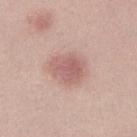Q: Was a biopsy performed?
A: imaged on a skin check; not biopsied
Q: Where on the body is the lesion?
A: the left lower leg
Q: What did automated image analysis measure?
A: an area of roughly 9.5 mm² and an eccentricity of roughly 0.55; a lesion-to-skin contrast of about 6.5 (normalized; higher = more distinct); an automated nevus-likeness rating near 80 out of 100
Q: Illumination type?
A: white-light
Q: How was this image acquired?
A: 15 mm crop, total-body photography
Q: What is the lesion's diameter?
A: ≈3.5 mm
Q: Patient demographics?
A: female, aged approximately 20